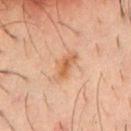This lesion was catalogued during total-body skin photography and was not selected for biopsy. The subject is a male aged approximately 35. The total-body-photography lesion software estimated a lesion area of about 6 mm² and a symmetry-axis asymmetry near 0.35. The software also gave a mean CIELAB color near L≈57 a*≈22 b*≈35, about 9 CIELAB-L* units darker than the surrounding skin, and a normalized lesion–skin contrast near 7. And it measured border irregularity of about 3.5 on a 0–10 scale, a color-variation rating of about 4.5/10, and radial color variation of about 1.5. The analysis additionally found a classifier nevus-likeness of about 30/100 and lesion-presence confidence of about 100/100. A close-up tile cropped from a whole-body skin photograph, about 15 mm across. The lesion is on the chest.